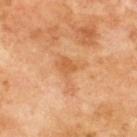Part of a total-body skin-imaging series; this lesion was reviewed on a skin check and was not flagged for biopsy. The patient is a male approximately 70 years of age. Cropped from a whole-body photographic skin survey; the tile spans about 15 mm. The lesion is located on the back.